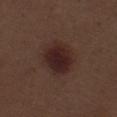Notes:
* workup — catalogued during a skin exam; not biopsied
* acquisition — ~15 mm tile from a whole-body skin photo
* lesion diameter — ≈4 mm
* tile lighting — white-light
* location — the left thigh
* patient — male, about 70 years old